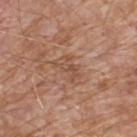Findings:
– biopsy status · catalogued during a skin exam; not biopsied
– site · the upper back
– lesion size · ~3.5 mm (longest diameter)
– automated metrics · an area of roughly 6.5 mm², an eccentricity of roughly 0.8, and two-axis asymmetry of about 0.4; a classifier nevus-likeness of about 0/100 and a lesion-detection confidence of about 100/100
– image source · 15 mm crop, total-body photography
– subject · male, roughly 80 years of age
– lighting · white-light illumination